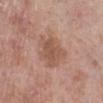Assessment: No biopsy was performed on this lesion — it was imaged during a full skin examination and was not determined to be concerning. Image and clinical context: A close-up tile cropped from a whole-body skin photograph, about 15 mm across. A female patient roughly 70 years of age. From the left lower leg.